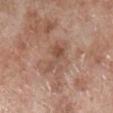Impression: The lesion was tiled from a total-body skin photograph and was not biopsied. Context: The recorded lesion diameter is about 3.5 mm. The lesion is on the right lower leg. Captured under white-light illumination. A male subject, about 70 years old. Automated tile analysis of the lesion measured a border-irregularity index near 7/10, internal color variation of about 2.5 on a 0–10 scale, and a peripheral color-asymmetry measure near 1. A 15 mm close-up extracted from a 3D total-body photography capture.